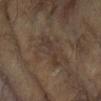Assessment:
No biopsy was performed on this lesion — it was imaged during a full skin examination and was not determined to be concerning.